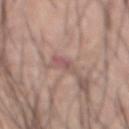A male subject, aged around 60. A 15 mm close-up tile from a total-body photography series done for melanoma screening. The tile uses white-light illumination. The total-body-photography lesion software estimated a lesion color around L≈54 a*≈22 b*≈21 in CIELAB and a lesion-to-skin contrast of about 6 (normalized; higher = more distinct). It also reported a border-irregularity index near 3/10 and a peripheral color-asymmetry measure near 0.5. The analysis additionally found a classifier nevus-likeness of about 0/100. On the front of the torso.